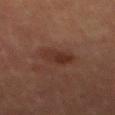Impression:
Recorded during total-body skin imaging; not selected for excision or biopsy.
Background:
The lesion-visualizer software estimated a mean CIELAB color near L≈27 a*≈19 b*≈23, about 6 CIELAB-L* units darker than the surrounding skin, and a lesion-to-skin contrast of about 7 (normalized; higher = more distinct). The analysis additionally found an automated nevus-likeness rating near 25 out of 100 and a lesion-detection confidence of about 100/100. A 15 mm close-up tile from a total-body photography series done for melanoma screening. Imaged with cross-polarized lighting. The subject is a male aged 63 to 67. Located on the front of the torso.